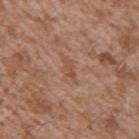workup = catalogued during a skin exam; not biopsied | TBP lesion metrics = a footprint of about 3.5 mm², an eccentricity of roughly 0.9, and a symmetry-axis asymmetry near 0.3; a border-irregularity rating of about 4/10, a within-lesion color-variation index near 1/10, and peripheral color asymmetry of about 0.5 | body site = the right upper arm | lighting = white-light illumination | lesion diameter = ~3 mm (longest diameter) | image = ~15 mm crop, total-body skin-cancer survey | patient = male, aged around 45.